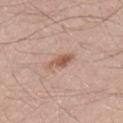biopsy status = no biopsy performed (imaged during a skin exam) | lighting = white-light illumination | anatomic site = the left thigh | lesion size = ≈3 mm | subject = male, in their mid- to late 40s | image = 15 mm crop, total-body photography.